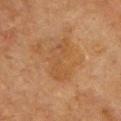workup = imaged on a skin check; not biopsied | image-analysis metrics = an area of roughly 12 mm², an eccentricity of roughly 0.85, and a symmetry-axis asymmetry near 0.35; border irregularity of about 4.5 on a 0–10 scale and a color-variation rating of about 3.5/10 | image = 15 mm crop, total-body photography | lighting = cross-polarized illumination | patient = male, aged 63–67 | size = ~5.5 mm (longest diameter) | site = the chest.